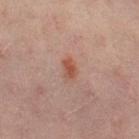Part of a total-body skin-imaging series; this lesion was reviewed on a skin check and was not flagged for biopsy.
About 2.5 mm across.
On the left thigh.
The tile uses cross-polarized illumination.
A female patient, aged around 50.
A 15 mm close-up extracted from a 3D total-body photography capture.